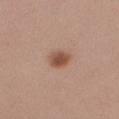Q: Was this lesion biopsied?
A: no biopsy performed (imaged during a skin exam)
Q: What is the imaging modality?
A: total-body-photography crop, ~15 mm field of view
Q: Lesion size?
A: about 2.5 mm
Q: Where on the body is the lesion?
A: the right forearm
Q: Patient demographics?
A: female, in their 40s